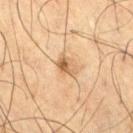Findings:
* workup · total-body-photography surveillance lesion; no biopsy
* illumination · cross-polarized illumination
* site · the leg
* image source · 15 mm crop, total-body photography
* subject · male, in their mid-60s
* image-analysis metrics · a lesion area of about 4.5 mm² and a shape eccentricity near 0.75; a lesion color around L≈49 a*≈15 b*≈31 in CIELAB, roughly 10 lightness units darker than nearby skin, and a lesion-to-skin contrast of about 7.5 (normalized; higher = more distinct); a border-irregularity index near 2.5/10, a within-lesion color-variation index near 6.5/10, and a peripheral color-asymmetry measure near 2.5